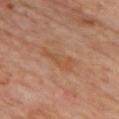Part of a total-body skin-imaging series; this lesion was reviewed on a skin check and was not flagged for biopsy. Automated tile analysis of the lesion measured an area of roughly 6.5 mm², an eccentricity of roughly 0.9, and a symmetry-axis asymmetry near 0.4. And it measured a mean CIELAB color near L≈50 a*≈21 b*≈32 and a normalized border contrast of about 5.5. From the mid back. About 4.5 mm across. A 15 mm close-up extracted from a 3D total-body photography capture. The patient is a male aged around 60.